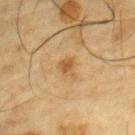Captured during whole-body skin photography for melanoma surveillance; the lesion was not biopsied.
A male patient, about 85 years old or older.
From the chest.
A roughly 15 mm field-of-view crop from a total-body skin photograph.